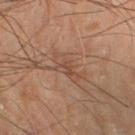Recorded during total-body skin imaging; not selected for excision or biopsy. A close-up tile cropped from a whole-body skin photograph, about 15 mm across. Approximately 4.5 mm at its widest. On the right thigh. The total-body-photography lesion software estimated an area of roughly 6.5 mm², an eccentricity of roughly 0.9, and a shape-asymmetry score of about 0.35 (0 = symmetric). And it measured a color-variation rating of about 2.5/10 and peripheral color asymmetry of about 0.5. The subject is a male aged around 65.